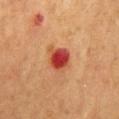Findings:
• patient — male, in their mid- to late 70s
• lesion diameter — ≈3 mm
• anatomic site — the mid back
• imaging modality — 15 mm crop, total-body photography